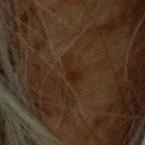Imaged during a routine full-body skin examination; the lesion was not biopsied and no histopathology is available.
The patient is a male aged 78–82.
This is a cross-polarized tile.
This image is a 15 mm lesion crop taken from a total-body photograph.
On the head or neck.
Automated image analysis of the tile measured a lesion color around L≈16 a*≈13 b*≈20 in CIELAB, a lesion–skin lightness drop of about 4, and a lesion-to-skin contrast of about 6.5 (normalized; higher = more distinct). The analysis additionally found a color-variation rating of about 1.5/10 and radial color variation of about 0.5. The software also gave a classifier nevus-likeness of about 0/100 and lesion-presence confidence of about 100/100.